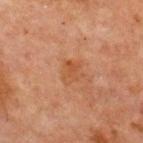The lesion was photographed on a routine skin check and not biopsied; there is no pathology result. Cropped from a total-body skin-imaging series; the visible field is about 15 mm. A male subject in their mid- to late 60s. Captured under cross-polarized illumination. On the front of the torso. The lesion's longest dimension is about 2.5 mm.